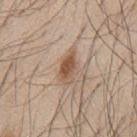This lesion was catalogued during total-body skin photography and was not selected for biopsy.
A male subject aged 43 to 47.
A 15 mm close-up extracted from a 3D total-body photography capture.
From the mid back.
Approximately 4 mm at its widest.